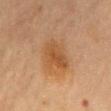Assessment: The lesion was tiled from a total-body skin photograph and was not biopsied. Context: The lesion is located on the back. A female subject, roughly 60 years of age. Imaged with cross-polarized lighting. Automated tile analysis of the lesion measured a shape eccentricity near 0.65 and a shape-asymmetry score of about 0.25 (0 = symmetric). The software also gave an average lesion color of about L≈46 a*≈19 b*≈35 (CIELAB) and roughly 7 lightness units darker than nearby skin. The software also gave border irregularity of about 3 on a 0–10 scale and a color-variation rating of about 3.5/10. The analysis additionally found a nevus-likeness score of about 90/100 and a lesion-detection confidence of about 100/100. A region of skin cropped from a whole-body photographic capture, roughly 15 mm wide. The lesion's longest dimension is about 5 mm.